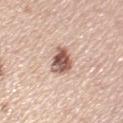biopsy status = catalogued during a skin exam; not biopsied